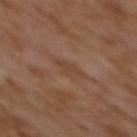biopsy status: catalogued during a skin exam; not biopsied
site: the upper back
subject: female, aged approximately 60
imaging modality: ~15 mm tile from a whole-body skin photo